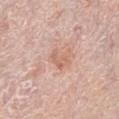Findings:
- biopsy status · catalogued during a skin exam; not biopsied
- subject · female, aged 63 to 67
- size · ≈2.5 mm
- body site · the left lower leg
- imaging modality · 15 mm crop, total-body photography